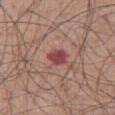notes = total-body-photography surveillance lesion; no biopsy.